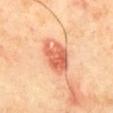Background:
The lesion is located on the mid back. The patient is a male roughly 65 years of age. Imaged with cross-polarized lighting. Cropped from a whole-body photographic skin survey; the tile spans about 15 mm. An algorithmic analysis of the crop reported an average lesion color of about L≈66 a*≈32 b*≈38 (CIELAB), a lesion–skin lightness drop of about 14, and a normalized border contrast of about 8.